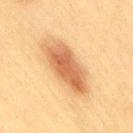Captured during whole-body skin photography for melanoma surveillance; the lesion was not biopsied.
Cropped from a total-body skin-imaging series; the visible field is about 15 mm.
The total-body-photography lesion software estimated a lesion area of about 20 mm², a shape eccentricity near 0.9, and a symmetry-axis asymmetry near 0.15.
Located on the right thigh.
A female subject aged around 50.
Longest diameter approximately 7.5 mm.